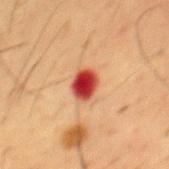Captured during whole-body skin photography for melanoma surveillance; the lesion was not biopsied. The lesion is located on the chest. A male subject aged around 55. Cropped from a whole-body photographic skin survey; the tile spans about 15 mm.